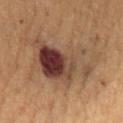The lesion was photographed on a routine skin check and not biopsied; there is no pathology result.
Located on the abdomen.
A female patient roughly 80 years of age.
Automated tile analysis of the lesion measured a lesion area of about 34 mm², an eccentricity of roughly 0.8, and two-axis asymmetry of about 0.35. The analysis additionally found a border-irregularity rating of about 5/10, internal color variation of about 10 on a 0–10 scale, and a peripheral color-asymmetry measure near 4.
Cropped from a total-body skin-imaging series; the visible field is about 15 mm.
The tile uses cross-polarized illumination.
Longest diameter approximately 9 mm.